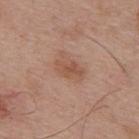biopsy status = no biopsy performed (imaged during a skin exam) | patient = male, approximately 55 years of age | acquisition = 15 mm crop, total-body photography | site = the back | tile lighting = white-light illumination.